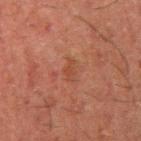Image and clinical context: Longest diameter approximately 2.5 mm. From the arm. A male patient aged 48 to 52. A 15 mm close-up extracted from a 3D total-body photography capture.